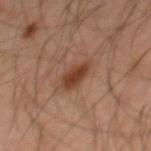Clinical impression: Imaged during a routine full-body skin examination; the lesion was not biopsied and no histopathology is available. Clinical summary: On the back. A close-up tile cropped from a whole-body skin photograph, about 15 mm across. Imaged with cross-polarized lighting. Approximately 4 mm at its widest. A male subject, aged 43–47.